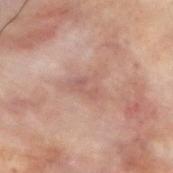| field | value |
|---|---|
| workup | imaged on a skin check; not biopsied |
| acquisition | 15 mm crop, total-body photography |
| patient | male, in their 30s |
| tile lighting | cross-polarized |
| site | the upper back |